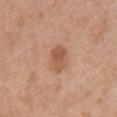Context: A female subject, aged around 40. The recorded lesion diameter is about 3.5 mm. Cropped from a total-body skin-imaging series; the visible field is about 15 mm. The lesion is located on the chest. An algorithmic analysis of the crop reported a lesion color around L≈56 a*≈22 b*≈33 in CIELAB, a lesion–skin lightness drop of about 10, and a lesion-to-skin contrast of about 7 (normalized; higher = more distinct).